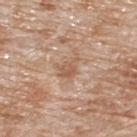The lesion was tiled from a total-body skin photograph and was not biopsied. The lesion-visualizer software estimated a nevus-likeness score of about 0/100 and lesion-presence confidence of about 100/100. A male patient, aged approximately 80. Located on the upper back. A 15 mm crop from a total-body photograph taken for skin-cancer surveillance.